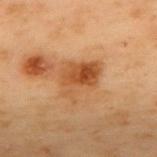The lesion was photographed on a routine skin check and not biopsied; there is no pathology result. From the upper back. The tile uses cross-polarized illumination. A 15 mm close-up tile from a total-body photography series done for melanoma screening. Longest diameter approximately 5 mm. A male patient, aged around 55.